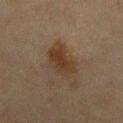No biopsy was performed on this lesion — it was imaged during a full skin examination and was not determined to be concerning. Located on the mid back. About 4 mm across. A region of skin cropped from a whole-body photographic capture, roughly 15 mm wide. This is a cross-polarized tile. A male patient about 70 years old.